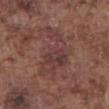No biopsy was performed on this lesion — it was imaged during a full skin examination and was not determined to be concerning. This image is a 15 mm lesion crop taken from a total-body photograph. Automated image analysis of the tile measured a mean CIELAB color near L≈38 a*≈20 b*≈20. The analysis additionally found border irregularity of about 8 on a 0–10 scale. It also reported an automated nevus-likeness rating near 5 out of 100. A male patient aged around 75. The lesion is located on the abdomen. The recorded lesion diameter is about 7.5 mm. The tile uses white-light illumination.